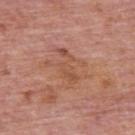Q: What are the patient's age and sex?
A: male, in their mid-70s
Q: What lighting was used for the tile?
A: white-light illumination
Q: Lesion location?
A: the back
Q: What is the imaging modality?
A: 15 mm crop, total-body photography